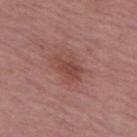Q: Was this lesion biopsied?
A: no biopsy performed (imaged during a skin exam)
Q: What is the imaging modality?
A: 15 mm crop, total-body photography
Q: Who is the patient?
A: female, aged 63–67
Q: Where on the body is the lesion?
A: the leg